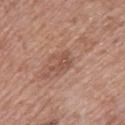Q: Was this lesion biopsied?
A: catalogued during a skin exam; not biopsied
Q: Lesion size?
A: about 3 mm
Q: Patient demographics?
A: female, roughly 60 years of age
Q: Illumination type?
A: white-light
Q: Lesion location?
A: the upper back
Q: Automated lesion metrics?
A: an area of roughly 4 mm², an eccentricity of roughly 0.8, and a symmetry-axis asymmetry near 0.5; a border-irregularity index near 5.5/10 and a within-lesion color-variation index near 1.5/10; a nevus-likeness score of about 0/100 and a lesion-detection confidence of about 100/100
Q: How was this image acquired?
A: total-body-photography crop, ~15 mm field of view